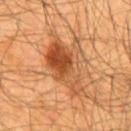Captured during whole-body skin photography for melanoma surveillance; the lesion was not biopsied. Located on the chest. The lesion's longest dimension is about 7.5 mm. A male subject in their 60s. A 15 mm close-up extracted from a 3D total-body photography capture. This is a cross-polarized tile.